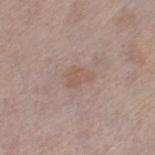Case summary:
• workup: imaged on a skin check; not biopsied
• image source: ~15 mm crop, total-body skin-cancer survey
• TBP lesion metrics: a lesion color around L≈55 a*≈16 b*≈25 in CIELAB and a lesion-to-skin contrast of about 5 (normalized; higher = more distinct); a border-irregularity rating of about 3.5/10, internal color variation of about 1.5 on a 0–10 scale, and peripheral color asymmetry of about 0.5; a classifier nevus-likeness of about 0/100 and lesion-presence confidence of about 100/100
• body site: the left thigh
• tile lighting: white-light
• lesion size: ~2.5 mm (longest diameter)
• patient: female, approximately 65 years of age